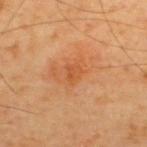Captured during whole-body skin photography for melanoma surveillance; the lesion was not biopsied.
Captured under cross-polarized illumination.
The lesion is located on the back.
A roughly 15 mm field-of-view crop from a total-body skin photograph.
Approximately 2 mm at its widest.
Automated tile analysis of the lesion measured a lesion area of about 3 mm², an outline eccentricity of about 0.5 (0 = round, 1 = elongated), and a symmetry-axis asymmetry near 0.3. And it measured border irregularity of about 2.5 on a 0–10 scale and a within-lesion color-variation index near 2/10.
A male subject about 55 years old.